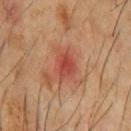Q: Was this lesion biopsied?
A: no biopsy performed (imaged during a skin exam)
Q: What is the anatomic site?
A: the front of the torso
Q: What are the patient's age and sex?
A: male, roughly 60 years of age
Q: What did automated image analysis measure?
A: an outline eccentricity of about 0.7 (0 = round, 1 = elongated) and a shape-asymmetry score of about 0.15 (0 = symmetric); roughly 9 lightness units darker than nearby skin; a border-irregularity rating of about 2/10, internal color variation of about 3.5 on a 0–10 scale, and peripheral color asymmetry of about 1; an automated nevus-likeness rating near 0 out of 100
Q: What is the imaging modality?
A: 15 mm crop, total-body photography